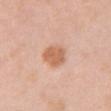No biopsy was performed on this lesion — it was imaged during a full skin examination and was not determined to be concerning.
The subject is a female aged 48 to 52.
Imaged with white-light lighting.
A close-up tile cropped from a whole-body skin photograph, about 15 mm across.
The lesion is located on the chest.
Measured at roughly 3.5 mm in maximum diameter.